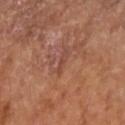The lesion was photographed on a routine skin check and not biopsied; there is no pathology result. A female subject, in their 70s. A roughly 15 mm field-of-view crop from a total-body skin photograph. The total-body-photography lesion software estimated a color-variation rating of about 0/10 and a peripheral color-asymmetry measure near 0. Approximately 3 mm at its widest. On the right forearm.